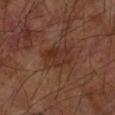Imaged with cross-polarized lighting. Measured at roughly 4 mm in maximum diameter. Automated image analysis of the tile measured a footprint of about 9.5 mm², an eccentricity of roughly 0.75, and two-axis asymmetry of about 0.15. The lesion is on the left forearm. A region of skin cropped from a whole-body photographic capture, roughly 15 mm wide. A male subject aged 68–72.